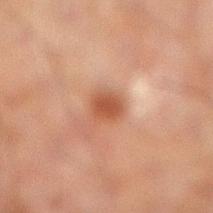* workup: catalogued during a skin exam; not biopsied
* TBP lesion metrics: an average lesion color of about L≈40 a*≈20 b*≈27 (CIELAB), a lesion–skin lightness drop of about 9, and a lesion-to-skin contrast of about 8 (normalized; higher = more distinct)
* diameter: ~2.5 mm (longest diameter)
* anatomic site: the left leg
* lighting: cross-polarized illumination
* image source: ~15 mm tile from a whole-body skin photo
* subject: male, in their 60s